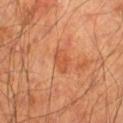• biopsy status: no biopsy performed (imaged during a skin exam)
• site: the right lower leg
• imaging modality: 15 mm crop, total-body photography
• patient: male, in their mid-60s
• automated lesion analysis: a footprint of about 4 mm² and two-axis asymmetry of about 0.3; a lesion color around L≈48 a*≈27 b*≈36 in CIELAB, a lesion–skin lightness drop of about 7, and a normalized border contrast of about 5.5; a within-lesion color-variation index near 1.5/10 and a peripheral color-asymmetry measure near 0.5; an automated nevus-likeness rating near 5 out of 100
• illumination: cross-polarized illumination